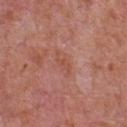notes: imaged on a skin check; not biopsied.